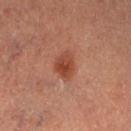Recorded during total-body skin imaging; not selected for excision or biopsy. The total-body-photography lesion software estimated a lesion color around L≈33 a*≈21 b*≈25 in CIELAB, roughly 8 lightness units darker than nearby skin, and a lesion-to-skin contrast of about 7.5 (normalized; higher = more distinct). The analysis additionally found internal color variation of about 3.5 on a 0–10 scale and radial color variation of about 1. Captured under cross-polarized illumination. Located on the left lower leg. The recorded lesion diameter is about 3 mm. The patient is a male aged around 60. A close-up tile cropped from a whole-body skin photograph, about 15 mm across.